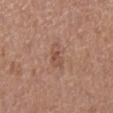notes: no biopsy performed (imaged during a skin exam) | lesion diameter: about 3 mm | image source: ~15 mm crop, total-body skin-cancer survey | body site: the right lower leg | subject: female, about 40 years old | automated lesion analysis: a lesion area of about 4 mm², a shape eccentricity near 0.8, and a shape-asymmetry score of about 0.4 (0 = symmetric); a detector confidence of about 100 out of 100 that the crop contains a lesion.